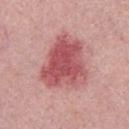Recorded during total-body skin imaging; not selected for excision or biopsy. A male patient aged 28 to 32. A close-up tile cropped from a whole-body skin photograph, about 15 mm across. About 6 mm across.